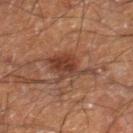<case>
<patient>
  <sex>male</sex>
  <age_approx>60</age_approx>
</patient>
<image>
  <source>total-body photography crop</source>
  <field_of_view_mm>15</field_of_view_mm>
</image>
<site>leg</site>
<lesion_size>
  <long_diameter_mm_approx>5.0</long_diameter_mm_approx>
</lesion_size>
<lighting>cross-polarized</lighting>
</case>